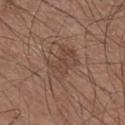biopsy_status: not biopsied; imaged during a skin examination
lighting: white-light
automated_metrics:
  cielab_L: 44
  cielab_a: 17
  cielab_b: 25
  vs_skin_darker_L: 6.0
  vs_skin_contrast_norm: 5.0
  nevus_likeness_0_100: 0
  lesion_detection_confidence_0_100: 100
site: right lower leg
image:
  source: total-body photography crop
  field_of_view_mm: 15
patient:
  sex: male
  age_approx: 75
lesion_size:
  long_diameter_mm_approx: 4.0